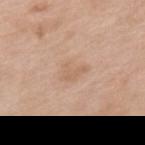workup=imaged on a skin check; not biopsied.